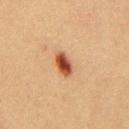This lesion was catalogued during total-body skin photography and was not selected for biopsy.
Captured under cross-polarized illumination.
The total-body-photography lesion software estimated an outline eccentricity of about 0.8 (0 = round, 1 = elongated) and a shape-asymmetry score of about 0.2 (0 = symmetric). The software also gave a border-irregularity rating of about 2/10, a within-lesion color-variation index near 5/10, and peripheral color asymmetry of about 1.5.
A 15 mm close-up extracted from a 3D total-body photography capture.
The patient is a male aged 38–42.
The recorded lesion diameter is about 3 mm.
The lesion is on the chest.